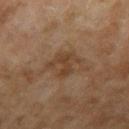Q: Was a biopsy performed?
A: catalogued during a skin exam; not biopsied
Q: Who is the patient?
A: female, in their 60s
Q: How was this image acquired?
A: ~15 mm tile from a whole-body skin photo
Q: Illumination type?
A: cross-polarized illumination
Q: Lesion location?
A: the arm
Q: Lesion size?
A: about 3 mm